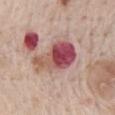Clinical impression: Imaged during a routine full-body skin examination; the lesion was not biopsied and no histopathology is available. Background: A roughly 15 mm field-of-view crop from a total-body skin photograph. Captured under white-light illumination. Measured at roughly 6.5 mm in maximum diameter. Located on the mid back. Automated tile analysis of the lesion measured an average lesion color of about L≈51 a*≈28 b*≈22 (CIELAB) and a lesion-to-skin contrast of about 11.5 (normalized; higher = more distinct). It also reported peripheral color asymmetry of about 5. It also reported an automated nevus-likeness rating near 0 out of 100 and lesion-presence confidence of about 100/100. The patient is a male in their mid- to late 60s.